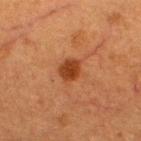<lesion>
  <biopsy_status>not biopsied; imaged during a skin examination</biopsy_status>
  <image>
    <source>total-body photography crop</source>
    <field_of_view_mm>15</field_of_view_mm>
  </image>
  <lesion_size>
    <long_diameter_mm_approx>3.0</long_diameter_mm_approx>
  </lesion_size>
  <patient>
    <sex>male</sex>
    <age_approx>50</age_approx>
  </patient>
  <automated_metrics>
    <border_irregularity_0_10>2.0</border_irregularity_0_10>
    <color_variation_0_10>2.0</color_variation_0_10>
    <nevus_likeness_0_100>95</nevus_likeness_0_100>
    <lesion_detection_confidence_0_100>100</lesion_detection_confidence_0_100>
  </automated_metrics>
  <lighting>cross-polarized</lighting>
  <site>upper back</site>
</lesion>